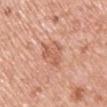Imaged during a routine full-body skin examination; the lesion was not biopsied and no histopathology is available.